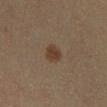– workup · no biopsy performed (imaged during a skin exam)
– image · ~15 mm crop, total-body skin-cancer survey
– site · the left lower leg
– patient · female, aged around 20
– diameter · ~2.5 mm (longest diameter)
– tile lighting · cross-polarized